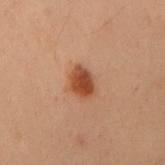- notes · total-body-photography surveillance lesion; no biopsy
- image · total-body-photography crop, ~15 mm field of view
- TBP lesion metrics · a lesion area of about 6 mm² and an eccentricity of roughly 0.65; a lesion color around L≈37 a*≈22 b*≈29 in CIELAB
- diameter · ≈3.5 mm
- location · the right upper arm
- subject · female, about 40 years old
- tile lighting · cross-polarized